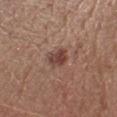notes: catalogued during a skin exam; not biopsied | site: the head or neck | imaging modality: 15 mm crop, total-body photography | subject: female, aged approximately 40 | automated metrics: a footprint of about 5 mm², an eccentricity of roughly 0.65, and a shape-asymmetry score of about 0.25 (0 = symmetric); internal color variation of about 3 on a 0–10 scale and radial color variation of about 1 | diameter: about 2.5 mm.